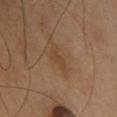Assessment:
The lesion was photographed on a routine skin check and not biopsied; there is no pathology result.
Clinical summary:
On the back. The lesion's longest dimension is about 3.5 mm. Imaged with cross-polarized lighting. An algorithmic analysis of the crop reported a lesion area of about 4 mm², a shape eccentricity near 0.9, and a symmetry-axis asymmetry near 0.55. And it measured a lesion–skin lightness drop of about 6. It also reported a border-irregularity rating of about 6.5/10 and radial color variation of about 0. It also reported a nevus-likeness score of about 0/100. The subject is a female aged around 40. A roughly 15 mm field-of-view crop from a total-body skin photograph.